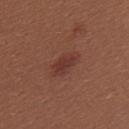Clinical impression: Part of a total-body skin-imaging series; this lesion was reviewed on a skin check and was not flagged for biopsy. Acquisition and patient details: On the back. Approximately 3.5 mm at its widest. A roughly 15 mm field-of-view crop from a total-body skin photograph. Imaged with white-light lighting. A female subject, roughly 25 years of age.